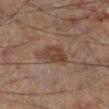Clinical impression: This lesion was catalogued during total-body skin photography and was not selected for biopsy. Clinical summary: A close-up tile cropped from a whole-body skin photograph, about 15 mm across. A male subject, approximately 60 years of age. From the left lower leg. Approximately 3.5 mm at its widest. This is a cross-polarized tile.